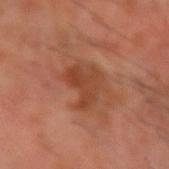| feature | finding |
|---|---|
| biopsy status | imaged on a skin check; not biopsied |
| size | ~4 mm (longest diameter) |
| subject | male, approximately 60 years of age |
| tile lighting | cross-polarized illumination |
| site | the right forearm |
| image source | ~15 mm tile from a whole-body skin photo |